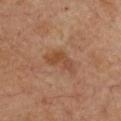Q: Is there a histopathology result?
A: catalogued during a skin exam; not biopsied
Q: What lighting was used for the tile?
A: cross-polarized
Q: What are the patient's age and sex?
A: female, aged approximately 60
Q: How large is the lesion?
A: about 4 mm
Q: How was this image acquired?
A: ~15 mm crop, total-body skin-cancer survey
Q: Where on the body is the lesion?
A: the chest